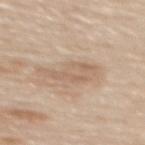Image and clinical context:
A female subject in their 60s. A roughly 15 mm field-of-view crop from a total-body skin photograph. Imaged with white-light lighting. The recorded lesion diameter is about 5.5 mm. From the upper back.